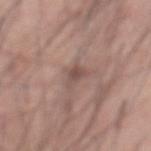Q: Is there a histopathology result?
A: total-body-photography surveillance lesion; no biopsy
Q: Lesion location?
A: the abdomen
Q: Illumination type?
A: white-light
Q: What is the imaging modality?
A: total-body-photography crop, ~15 mm field of view
Q: What are the patient's age and sex?
A: male, about 60 years old